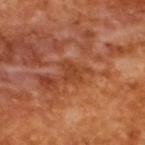This lesion was catalogued during total-body skin photography and was not selected for biopsy. A 15 mm close-up tile from a total-body photography series done for melanoma screening. A male patient, aged approximately 65. Measured at roughly 3.5 mm in maximum diameter.